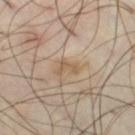Case summary:
• biopsy status — catalogued during a skin exam; not biopsied
• tile lighting — cross-polarized
• acquisition — 15 mm crop, total-body photography
• body site — the right thigh
• subject — male, approximately 40 years of age
• lesion diameter — about 2.5 mm